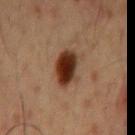notes: imaged on a skin check; not biopsied
diameter: about 4.5 mm
location: the chest
imaging modality: 15 mm crop, total-body photography
patient: male, aged around 50
lighting: cross-polarized